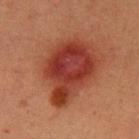notes: total-body-photography surveillance lesion; no biopsy | location: the left upper arm | patient: male, about 40 years old | acquisition: total-body-photography crop, ~15 mm field of view | tile lighting: cross-polarized illumination.